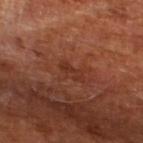| field | value |
|---|---|
| follow-up | no biopsy performed (imaged during a skin exam) |
| imaging modality | 15 mm crop, total-body photography |
| lesion diameter | ≈3 mm |
| subject | about 65 years old |
| body site | the left lower leg |